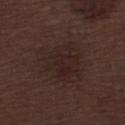A 15 mm close-up tile from a total-body photography series done for melanoma screening.
From the left lower leg.
A male patient, aged 68–72.